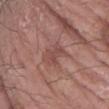Notes:
* workup — total-body-photography surveillance lesion; no biopsy
* subject — male, aged 58–62
* acquisition — ~15 mm crop, total-body skin-cancer survey
* site — the right forearm